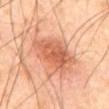No biopsy was performed on this lesion — it was imaged during a full skin examination and was not determined to be concerning. About 6 mm across. The tile uses cross-polarized illumination. Located on the abdomen. A male subject aged approximately 65. This image is a 15 mm lesion crop taken from a total-body photograph.